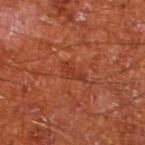Findings:
• workup · imaged on a skin check; not biopsied
• illumination · cross-polarized illumination
• diameter · ≈3 mm
• image · 15 mm crop, total-body photography
• patient · male, in their mid-60s
• location · the left lower leg